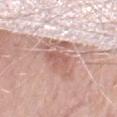On the right thigh. The lesion-visualizer software estimated a border-irregularity rating of about 4.5/10, a color-variation rating of about 5/10, and peripheral color asymmetry of about 1.5. Captured under white-light illumination. A lesion tile, about 15 mm wide, cut from a 3D total-body photograph. A male subject roughly 80 years of age.